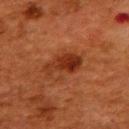Impression: The lesion was photographed on a routine skin check and not biopsied; there is no pathology result. Background: The total-body-photography lesion software estimated a footprint of about 9 mm² and an outline eccentricity of about 0.85 (0 = round, 1 = elongated). The software also gave internal color variation of about 5 on a 0–10 scale and radial color variation of about 1.5. A female subject about 50 years old. About 4.5 mm across. The tile uses cross-polarized illumination. A 15 mm crop from a total-body photograph taken for skin-cancer surveillance. The lesion is on the upper back.